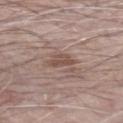No biopsy was performed on this lesion — it was imaged during a full skin examination and was not determined to be concerning. On the left forearm. An algorithmic analysis of the crop reported a footprint of about 4 mm², an outline eccentricity of about 0.8 (0 = round, 1 = elongated), and a shape-asymmetry score of about 0.3 (0 = symmetric). The software also gave an average lesion color of about L≈50 a*≈17 b*≈23 (CIELAB) and roughly 8 lightness units darker than nearby skin. And it measured border irregularity of about 3 on a 0–10 scale, a within-lesion color-variation index near 2.5/10, and a peripheral color-asymmetry measure near 1. A region of skin cropped from a whole-body photographic capture, roughly 15 mm wide. The patient is a male in their 70s. About 3 mm across. The tile uses white-light illumination.